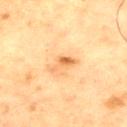Case summary:
– follow-up: imaged on a skin check; not biopsied
– size: ~3.5 mm (longest diameter)
– body site: the front of the torso
– patient: male, roughly 65 years of age
– tile lighting: cross-polarized
– automated lesion analysis: an average lesion color of about L≈56 a*≈20 b*≈38 (CIELAB) and a normalized border contrast of about 7; a classifier nevus-likeness of about 40/100
– imaging modality: 15 mm crop, total-body photography